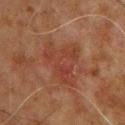workup: total-body-photography surveillance lesion; no biopsy | subject: male, roughly 60 years of age | illumination: cross-polarized | anatomic site: the chest | imaging modality: total-body-photography crop, ~15 mm field of view | size: about 5.5 mm.